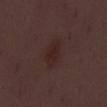Case summary:
– biopsy status: imaged on a skin check; not biopsied
– image: ~15 mm crop, total-body skin-cancer survey
– TBP lesion metrics: an area of roughly 5.5 mm² and a symmetry-axis asymmetry near 0.25; a lesion-to-skin contrast of about 6.5 (normalized; higher = more distinct); a border-irregularity index near 2.5/10 and a peripheral color-asymmetry measure near 0.5
– tile lighting: white-light illumination
– subject: male, approximately 50 years of age